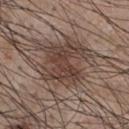notes = imaged on a skin check; not biopsied | lesion size = ~4.5 mm (longest diameter) | subject = male, aged around 55 | body site = the front of the torso | imaging modality = ~15 mm tile from a whole-body skin photo.